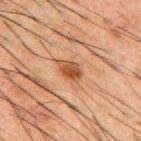This lesion was catalogued during total-body skin photography and was not selected for biopsy.
A region of skin cropped from a whole-body photographic capture, roughly 15 mm wide.
An algorithmic analysis of the crop reported a footprint of about 4.5 mm², an eccentricity of roughly 0.75, and two-axis asymmetry of about 0.25. And it measured about 10 CIELAB-L* units darker than the surrounding skin. And it measured a border-irregularity rating of about 2/10, a color-variation rating of about 4/10, and peripheral color asymmetry of about 1.5.
The tile uses cross-polarized illumination.
A male patient in their 50s.
From the mid back.